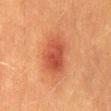biopsy status: total-body-photography surveillance lesion; no biopsy | image source: ~15 mm tile from a whole-body skin photo | subject: male, aged 38–42 | size: ~5 mm (longest diameter) | body site: the mid back | illumination: cross-polarized illumination.